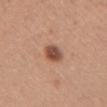Case summary:
• notes — imaged on a skin check; not biopsied
• patient — female, approximately 35 years of age
• acquisition — total-body-photography crop, ~15 mm field of view
• automated metrics — an average lesion color of about L≈50 a*≈23 b*≈30 (CIELAB), about 14 CIELAB-L* units darker than the surrounding skin, and a normalized lesion–skin contrast near 9.5; a color-variation rating of about 5/10 and peripheral color asymmetry of about 1.5; a nevus-likeness score of about 95/100
• location — the chest
• lighting — white-light
• lesion size — ~3 mm (longest diameter)